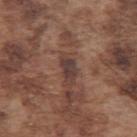acquisition: ~15 mm tile from a whole-body skin photo
location: the left upper arm
lesion diameter: ≈3 mm
subject: male, aged 73–77